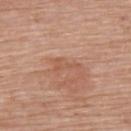follow-up: imaged on a skin check; not biopsied
automated metrics: a footprint of about 3 mm², a shape eccentricity near 0.95, and a shape-asymmetry score of about 0.7 (0 = symmetric); a lesion color around L≈55 a*≈23 b*≈32 in CIELAB and a normalized lesion–skin contrast near 4.5
site: the upper back
imaging modality: ~15 mm crop, total-body skin-cancer survey
lesion size: ~3.5 mm (longest diameter)
illumination: white-light illumination
subject: female, roughly 65 years of age